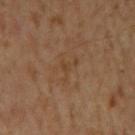Assessment:
The lesion was photographed on a routine skin check and not biopsied; there is no pathology result.
Background:
Longest diameter approximately 3 mm. The lesion is on the mid back. A 15 mm close-up tile from a total-body photography series done for melanoma screening. A male patient, aged 58 to 62.